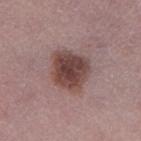No biopsy was performed on this lesion — it was imaged during a full skin examination and was not determined to be concerning.
The subject is a female approximately 50 years of age.
Approximately 4.5 mm at its widest.
A 15 mm close-up tile from a total-body photography series done for melanoma screening.
From the leg.
The tile uses white-light illumination.